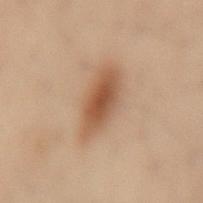Impression: This lesion was catalogued during total-body skin photography and was not selected for biopsy. Acquisition and patient details: Automated tile analysis of the lesion measured an eccentricity of roughly 0.85 and two-axis asymmetry of about 0.2. And it measured a nevus-likeness score of about 95/100 and lesion-presence confidence of about 100/100. A close-up tile cropped from a whole-body skin photograph, about 15 mm across. A female subject, aged 53 to 57. On the mid back. The tile uses cross-polarized illumination. Approximately 5.5 mm at its widest.